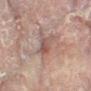Captured during whole-body skin photography for melanoma surveillance; the lesion was not biopsied.
A 15 mm crop from a total-body photograph taken for skin-cancer surveillance.
The tile uses cross-polarized illumination.
A female patient, approximately 80 years of age.
From the right leg.